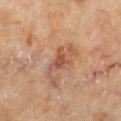Part of a total-body skin-imaging series; this lesion was reviewed on a skin check and was not flagged for biopsy.
A female subject, aged around 60.
About 3.5 mm across.
The total-body-photography lesion software estimated a border-irregularity rating of about 5.5/10 and a peripheral color-asymmetry measure near 1.5.
The tile uses cross-polarized illumination.
The lesion is on the leg.
A region of skin cropped from a whole-body photographic capture, roughly 15 mm wide.